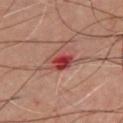patient — male, roughly 50 years of age; site — the chest; image-analysis metrics — a within-lesion color-variation index near 8/10; lesion size — about 3 mm; imaging modality — ~15 mm tile from a whole-body skin photo.